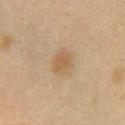Impression:
Recorded during total-body skin imaging; not selected for excision or biopsy.
Acquisition and patient details:
Located on the chest. A 15 mm close-up extracted from a 3D total-body photography capture. The patient is a female aged around 40.